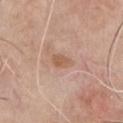The lesion was tiled from a total-body skin photograph and was not biopsied.
A roughly 15 mm field-of-view crop from a total-body skin photograph.
The lesion's longest dimension is about 3 mm.
Located on the chest.
The tile uses white-light illumination.
A male patient, roughly 75 years of age.